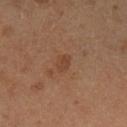follow-up — total-body-photography surveillance lesion; no biopsy | anatomic site — the left lower leg | patient — male, aged 58 to 62 | image — 15 mm crop, total-body photography.